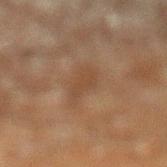  patient:
    sex: male
    age_approx: 60
  lesion_size:
    long_diameter_mm_approx: 5.0
  image:
    source: total-body photography crop
    field_of_view_mm: 15
  site: left lower leg